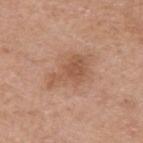No biopsy was performed on this lesion — it was imaged during a full skin examination and was not determined to be concerning. A male patient approximately 65 years of age. On the arm. Captured under white-light illumination. A 15 mm close-up tile from a total-body photography series done for melanoma screening. Longest diameter approximately 5.5 mm.